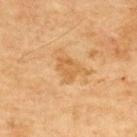Impression:
Recorded during total-body skin imaging; not selected for excision or biopsy.
Background:
Located on the upper back. A male subject in their 70s. An algorithmic analysis of the crop reported a footprint of about 5.5 mm², an outline eccentricity of about 0.6 (0 = round, 1 = elongated), and two-axis asymmetry of about 0.35. A close-up tile cropped from a whole-body skin photograph, about 15 mm across.